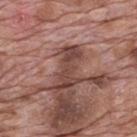Impression:
Captured during whole-body skin photography for melanoma surveillance; the lesion was not biopsied.
Acquisition and patient details:
This is a white-light tile. On the mid back. The patient is a male approximately 70 years of age. A region of skin cropped from a whole-body photographic capture, roughly 15 mm wide.